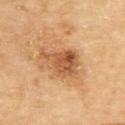biopsy_status: not biopsied; imaged during a skin examination
site: upper back
lighting: cross-polarized
automated_metrics:
  cielab_L: 53
  cielab_a: 23
  cielab_b: 38
  nevus_likeness_0_100: 85
  lesion_detection_confidence_0_100: 100
image:
  source: total-body photography crop
  field_of_view_mm: 15
patient:
  sex: male
  age_approx: 85+
lesion_size:
  long_diameter_mm_approx: 4.5